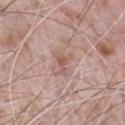Assessment:
The lesion was tiled from a total-body skin photograph and was not biopsied.
Context:
The lesion is on the chest. This is a white-light tile. Cropped from a total-body skin-imaging series; the visible field is about 15 mm. A male subject aged 68–72.